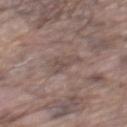The lesion was tiled from a total-body skin photograph and was not biopsied. On the mid back. A 15 mm close-up tile from a total-body photography series done for melanoma screening. The patient is a male about 75 years old.